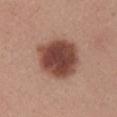| feature | finding |
|---|---|
| notes | catalogued during a skin exam; not biopsied |
| lighting | white-light illumination |
| site | the mid back |
| lesion size | ≈5.5 mm |
| imaging modality | ~15 mm crop, total-body skin-cancer survey |
| patient | female, about 25 years old |